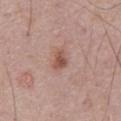Findings:
• workup · imaged on a skin check; not biopsied
• site · the abdomen
• image · ~15 mm crop, total-body skin-cancer survey
• subject · male, aged around 70
• image-analysis metrics · a lesion area of about 3.5 mm² and a shape eccentricity near 0.75; a lesion color around L≈52 a*≈22 b*≈26 in CIELAB, roughly 11 lightness units darker than nearby skin, and a lesion-to-skin contrast of about 8 (normalized; higher = more distinct); an automated nevus-likeness rating near 70 out of 100 and a detector confidence of about 100 out of 100 that the crop contains a lesion
• lesion diameter · ≈2.5 mm
• tile lighting · white-light